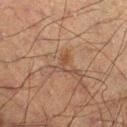workup: no biopsy performed (imaged during a skin exam)
image: ~15 mm tile from a whole-body skin photo
anatomic site: the right lower leg
automated lesion analysis: a lesion area of about 2.5 mm² and two-axis asymmetry of about 0.5; a border-irregularity rating of about 5.5/10, internal color variation of about 0 on a 0–10 scale, and peripheral color asymmetry of about 0
size: ~2.5 mm (longest diameter)
lighting: cross-polarized illumination
patient: male, aged approximately 50